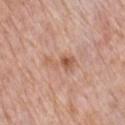Assessment:
This lesion was catalogued during total-body skin photography and was not selected for biopsy.
Clinical summary:
Cropped from a total-body skin-imaging series; the visible field is about 15 mm. Longest diameter approximately 4 mm. From the chest. An algorithmic analysis of the crop reported a footprint of about 5.5 mm², a shape eccentricity near 0.9, and a shape-asymmetry score of about 0.55 (0 = symmetric). And it measured a lesion color around L≈58 a*≈22 b*≈31 in CIELAB, a lesion–skin lightness drop of about 9, and a normalized lesion–skin contrast near 6.5. The patient is a male aged around 60. Captured under white-light illumination.